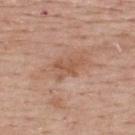<tbp_lesion>
  <biopsy_status>not biopsied; imaged during a skin examination</biopsy_status>
  <site>upper back</site>
  <patient>
    <sex>female</sex>
    <age_approx>65</age_approx>
  </patient>
  <image>
    <source>total-body photography crop</source>
    <field_of_view_mm>15</field_of_view_mm>
  </image>
</tbp_lesion>